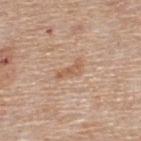workup: imaged on a skin check; not biopsied
patient: male, in their mid-50s
illumination: white-light
lesion diameter: ≈3 mm
imaging modality: 15 mm crop, total-body photography
body site: the back